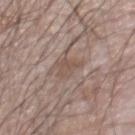notes: no biopsy performed (imaged during a skin exam) | patient: male, in their 70s | TBP lesion metrics: border irregularity of about 4.5 on a 0–10 scale and internal color variation of about 2 on a 0–10 scale; a classifier nevus-likeness of about 0/100 and lesion-presence confidence of about 60/100 | image source: total-body-photography crop, ~15 mm field of view | location: the chest.